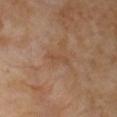Impression: The lesion was tiled from a total-body skin photograph and was not biopsied. Clinical summary: Located on the chest. A 15 mm close-up tile from a total-body photography series done for melanoma screening. A female subject, roughly 55 years of age. Captured under cross-polarized illumination. Longest diameter approximately 3 mm.